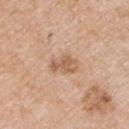Recorded during total-body skin imaging; not selected for excision or biopsy.
A region of skin cropped from a whole-body photographic capture, roughly 15 mm wide.
An algorithmic analysis of the crop reported a lesion color around L≈59 a*≈20 b*≈32 in CIELAB, roughly 10 lightness units darker than nearby skin, and a lesion-to-skin contrast of about 6.5 (normalized; higher = more distinct).
Located on the right upper arm.
The subject is a male in their 50s.
Captured under white-light illumination.